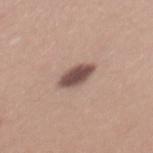• notes: imaged on a skin check; not biopsied
• patient: female, about 25 years old
• image source: 15 mm crop, total-body photography
• anatomic site: the mid back